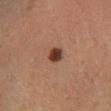| field | value |
|---|---|
| follow-up | total-body-photography surveillance lesion; no biopsy |
| subject | female, aged approximately 60 |
| image source | 15 mm crop, total-body photography |
| image-analysis metrics | a within-lesion color-variation index near 4.5/10 and radial color variation of about 1.5 |
| anatomic site | the right lower leg |